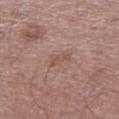Q: Was this lesion biopsied?
A: no biopsy performed (imaged during a skin exam)
Q: Where on the body is the lesion?
A: the left lower leg
Q: How was this image acquired?
A: 15 mm crop, total-body photography
Q: How was the tile lit?
A: white-light illumination
Q: How large is the lesion?
A: ~2.5 mm (longest diameter)
Q: Patient demographics?
A: male, aged 58 to 62